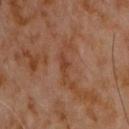Q: Was this lesion biopsied?
A: total-body-photography surveillance lesion; no biopsy
Q: Where on the body is the lesion?
A: the chest
Q: What is the imaging modality?
A: 15 mm crop, total-body photography
Q: What is the lesion's diameter?
A: ≈3 mm
Q: Patient demographics?
A: male, approximately 60 years of age
Q: How was the tile lit?
A: cross-polarized